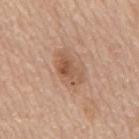Part of a total-body skin-imaging series; this lesion was reviewed on a skin check and was not flagged for biopsy. The lesion is located on the back. A 15 mm close-up extracted from a 3D total-body photography capture. The total-body-photography lesion software estimated a footprint of about 7.5 mm² and an eccentricity of roughly 0.8. The software also gave a mean CIELAB color near L≈55 a*≈20 b*≈32, roughly 10 lightness units darker than nearby skin, and a normalized lesion–skin contrast near 7. And it measured border irregularity of about 4 on a 0–10 scale, internal color variation of about 6 on a 0–10 scale, and peripheral color asymmetry of about 2. The analysis additionally found a classifier nevus-likeness of about 35/100 and a lesion-detection confidence of about 100/100. A male subject approximately 65 years of age.